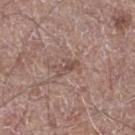biopsy status: total-body-photography surveillance lesion; no biopsy
tile lighting: white-light illumination
body site: the leg
subject: male, in their 70s
lesion size: ~3 mm (longest diameter)
image source: ~15 mm crop, total-body skin-cancer survey